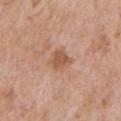{"biopsy_status": "not biopsied; imaged during a skin examination", "lesion_size": {"long_diameter_mm_approx": 3.0}, "automated_metrics": {"nevus_likeness_0_100": 10, "lesion_detection_confidence_0_100": 100}, "site": "front of the torso", "patient": {"sex": "male", "age_approx": 60}, "image": {"source": "total-body photography crop", "field_of_view_mm": 15}, "lighting": "white-light"}